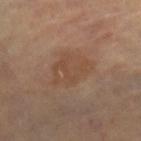follow-up: total-body-photography surveillance lesion; no biopsy | automated metrics: a shape eccentricity near 0.55 and a symmetry-axis asymmetry near 0.25; an automated nevus-likeness rating near 0 out of 100 and a lesion-detection confidence of about 100/100 | patient: female, aged 63–67 | location: the right lower leg | image: 15 mm crop, total-body photography | illumination: cross-polarized illumination.